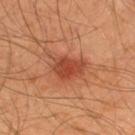follow-up: catalogued during a skin exam; not biopsied | acquisition: ~15 mm crop, total-body skin-cancer survey | patient: male, aged around 45 | size: ≈5 mm | site: the upper back.